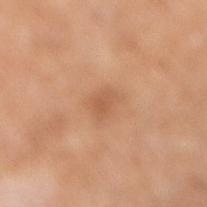biopsy status=no biopsy performed (imaged during a skin exam); subject=male, roughly 65 years of age; location=the right lower leg; image=15 mm crop, total-body photography; lesion size=about 2.5 mm; illumination=cross-polarized illumination.